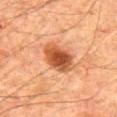Captured during whole-body skin photography for melanoma surveillance; the lesion was not biopsied.
The lesion is located on the abdomen.
The lesion's longest dimension is about 5 mm.
The tile uses cross-polarized illumination.
The patient is a male aged 78–82.
This image is a 15 mm lesion crop taken from a total-body photograph.